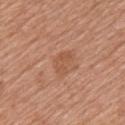Notes:
- notes: total-body-photography surveillance lesion; no biopsy
- patient: male, aged 58–62
- body site: the chest
- lesion diameter: about 3 mm
- lighting: white-light illumination
- acquisition: ~15 mm crop, total-body skin-cancer survey